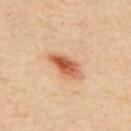follow-up = no biopsy performed (imaged during a skin exam) | imaging modality = ~15 mm tile from a whole-body skin photo | subject = male, aged 38–42 | lighting = cross-polarized illumination | size = about 4.5 mm | anatomic site = the upper back.